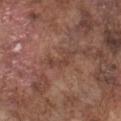Clinical impression:
Part of a total-body skin-imaging series; this lesion was reviewed on a skin check and was not flagged for biopsy.
Acquisition and patient details:
The lesion is located on the chest. This image is a 15 mm lesion crop taken from a total-body photograph. Captured under white-light illumination. A male patient aged approximately 75.